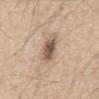| field | value |
|---|---|
| follow-up | imaged on a skin check; not biopsied |
| lesion size | ~4 mm (longest diameter) |
| location | the front of the torso |
| imaging modality | total-body-photography crop, ~15 mm field of view |
| patient | male, aged 63–67 |